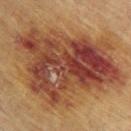The lesion was photographed on a routine skin check and not biopsied; there is no pathology result.
This is a cross-polarized tile.
A 15 mm close-up extracted from a 3D total-body photography capture.
The lesion is on the upper back.
A female patient aged around 80.